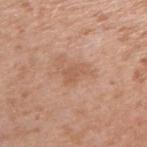workup — imaged on a skin check; not biopsied
illumination — white-light illumination
TBP lesion metrics — a lesion color around L≈57 a*≈22 b*≈32 in CIELAB, roughly 7 lightness units darker than nearby skin, and a normalized border contrast of about 5; border irregularity of about 3 on a 0–10 scale and a peripheral color-asymmetry measure near 0.5
body site — the right upper arm
subject — female, aged around 40
size — ≈2.5 mm
image — total-body-photography crop, ~15 mm field of view